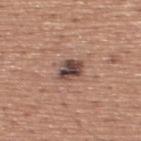notes: total-body-photography surveillance lesion; no biopsy
subject: male, aged 43–47
imaging modality: 15 mm crop, total-body photography
lesion diameter: ≈3 mm
location: the upper back
lighting: white-light illumination